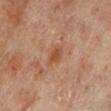Recorded during total-body skin imaging; not selected for excision or biopsy. The lesion is on the left lower leg. A lesion tile, about 15 mm wide, cut from a 3D total-body photograph. A female patient, roughly 80 years of age. About 3 mm across. Automated tile analysis of the lesion measured an area of roughly 4.5 mm², a shape eccentricity near 0.7, and a symmetry-axis asymmetry near 0.2. The analysis additionally found a mean CIELAB color near L≈42 a*≈21 b*≈30 and a lesion-to-skin contrast of about 7 (normalized; higher = more distinct). The analysis additionally found a detector confidence of about 100 out of 100 that the crop contains a lesion. Captured under cross-polarized illumination.